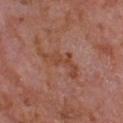Q: Was a biopsy performed?
A: no biopsy performed (imaged during a skin exam)
Q: How was the tile lit?
A: white-light illumination
Q: Where on the body is the lesion?
A: the chest
Q: How large is the lesion?
A: ~5.5 mm (longest diameter)
Q: How was this image acquired?
A: ~15 mm crop, total-body skin-cancer survey
Q: Who is the patient?
A: male, aged 63–67